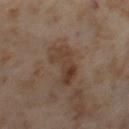The lesion was tiled from a total-body skin photograph and was not biopsied.
The lesion is located on the left thigh.
The patient is a female aged 53 to 57.
The recorded lesion diameter is about 6 mm.
A 15 mm crop from a total-body photograph taken for skin-cancer surveillance.
This is a cross-polarized tile.